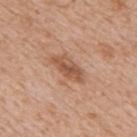Clinical summary:
The patient is a male roughly 65 years of age. Automated image analysis of the tile measured border irregularity of about 3 on a 0–10 scale, internal color variation of about 4 on a 0–10 scale, and a peripheral color-asymmetry measure near 1.5. The lesion is located on the upper back. A lesion tile, about 15 mm wide, cut from a 3D total-body photograph.